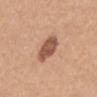biopsy status: imaged on a skin check; not biopsied | anatomic site: the mid back | tile lighting: white-light illumination | acquisition: total-body-photography crop, ~15 mm field of view | size: about 3.5 mm | patient: female, in their mid- to late 50s.